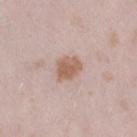biopsy status: total-body-photography surveillance lesion; no biopsy
imaging modality: ~15 mm tile from a whole-body skin photo
subject: female, aged 23 to 27
illumination: white-light
size: ≈3 mm
automated metrics: a shape eccentricity near 0.65 and two-axis asymmetry of about 0.2; a lesion color around L≈59 a*≈19 b*≈27 in CIELAB, a lesion–skin lightness drop of about 11, and a lesion-to-skin contrast of about 8.5 (normalized; higher = more distinct); a border-irregularity rating of about 2/10 and a color-variation rating of about 2.5/10; a classifier nevus-likeness of about 90/100
anatomic site: the leg